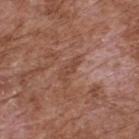Assessment:
The lesion was tiled from a total-body skin photograph and was not biopsied.
Image and clinical context:
The recorded lesion diameter is about 3 mm. A 15 mm close-up extracted from a 3D total-body photography capture. On the upper back. The subject is a male in their mid-70s. The tile uses white-light illumination.